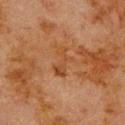No biopsy was performed on this lesion — it was imaged during a full skin examination and was not determined to be concerning. The patient is a male aged 78 to 82. Cropped from a total-body skin-imaging series; the visible field is about 15 mm. The recorded lesion diameter is about 4 mm. Imaged with cross-polarized lighting. From the upper back.